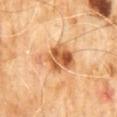A 15 mm close-up tile from a total-body photography series done for melanoma screening.
From the abdomen.
This is a cross-polarized tile.
A male subject, in their 60s.